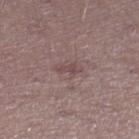The subject is a male in their 40s. A lesion tile, about 15 mm wide, cut from a 3D total-body photograph. On the right lower leg.